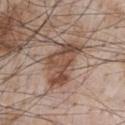Captured during whole-body skin photography for melanoma surveillance; the lesion was not biopsied. Cropped from a total-body skin-imaging series; the visible field is about 15 mm. The patient is a male in their mid-60s. The lesion's longest dimension is about 6 mm. On the chest. The tile uses white-light illumination.